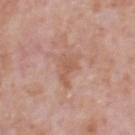Assessment:
Recorded during total-body skin imaging; not selected for excision or biopsy.
Image and clinical context:
A close-up tile cropped from a whole-body skin photograph, about 15 mm across. Located on the head or neck. A male subject roughly 70 years of age. Measured at roughly 3.5 mm in maximum diameter. Captured under white-light illumination.